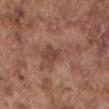Case summary:
* notes: total-body-photography surveillance lesion; no biopsy
* automated lesion analysis: a symmetry-axis asymmetry near 0.65; a mean CIELAB color near L≈46 a*≈21 b*≈27, roughly 6 lightness units darker than nearby skin, and a normalized lesion–skin contrast near 5; a border-irregularity rating of about 10/10 and internal color variation of about 2.5 on a 0–10 scale; an automated nevus-likeness rating near 0 out of 100
* patient: male, aged around 75
* image source: total-body-photography crop, ~15 mm field of view
* lighting: white-light illumination
* site: the back